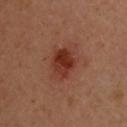This image is a 15 mm lesion crop taken from a total-body photograph. The lesion is on the upper back. Captured under cross-polarized illumination. Approximately 4.5 mm at its widest. A female subject, aged 38–42.